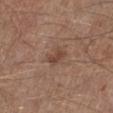• biopsy status — total-body-photography surveillance lesion; no biopsy
• lesion size — ≈3 mm
• acquisition — 15 mm crop, total-body photography
• lighting — white-light
• automated lesion analysis — a detector confidence of about 100 out of 100 that the crop contains a lesion
• anatomic site — the left lower leg
• patient — male, about 55 years old